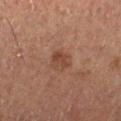A male patient roughly 60 years of age. A close-up tile cropped from a whole-body skin photograph, about 15 mm across. The tile uses cross-polarized illumination. The total-body-photography lesion software estimated a lesion area of about 5 mm² and two-axis asymmetry of about 0.2. It also reported a lesion color around L≈33 a*≈17 b*≈23 in CIELAB and roughly 6 lightness units darker than nearby skin. And it measured a classifier nevus-likeness of about 20/100 and a detector confidence of about 100 out of 100 that the crop contains a lesion. From the left lower leg. Measured at roughly 2.5 mm in maximum diameter.